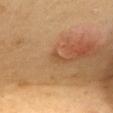notes=total-body-photography surveillance lesion; no biopsy
subject=female, aged 58–62
diameter=~1.5 mm (longest diameter)
body site=the mid back
imaging modality=~15 mm tile from a whole-body skin photo
tile lighting=cross-polarized